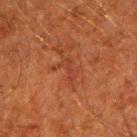  biopsy_status: not biopsied; imaged during a skin examination
  site: right upper arm
  image:
    source: total-body photography crop
    field_of_view_mm: 15
  patient:
    sex: male
    age_approx: 60